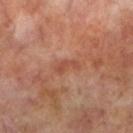On the left lower leg.
Approximately 3 mm at its widest.
The tile uses cross-polarized illumination.
Cropped from a whole-body photographic skin survey; the tile spans about 15 mm.
A male subject, aged approximately 70.
Automated tile analysis of the lesion measured a lesion area of about 3.5 mm², an eccentricity of roughly 0.9, and a shape-asymmetry score of about 0.5 (0 = symmetric). It also reported a mean CIELAB color near L≈48 a*≈25 b*≈31, roughly 7 lightness units darker than nearby skin, and a normalized lesion–skin contrast near 5.5. And it measured a border-irregularity index near 5/10, a color-variation rating of about 1/10, and a peripheral color-asymmetry measure near 0. The software also gave a classifier nevus-likeness of about 0/100 and lesion-presence confidence of about 100/100.